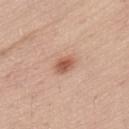Captured during whole-body skin photography for melanoma surveillance; the lesion was not biopsied. From the lower back. A male patient, roughly 55 years of age. A roughly 15 mm field-of-view crop from a total-body skin photograph.